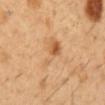Notes:
- notes — no biopsy performed (imaged during a skin exam)
- image — ~15 mm tile from a whole-body skin photo
- patient — male, aged 48–52
- location — the abdomen